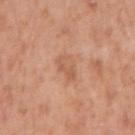Case summary:
– biopsy status — catalogued during a skin exam; not biopsied
– image source — ~15 mm tile from a whole-body skin photo
– illumination — white-light
– automated lesion analysis — an area of roughly 6 mm², an eccentricity of roughly 0.8, and a symmetry-axis asymmetry near 0.25; a lesion–skin lightness drop of about 7 and a normalized border contrast of about 5
– subject — male, approximately 50 years of age
– location — the right upper arm
– size — about 3.5 mm